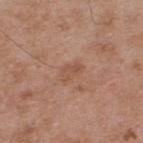  biopsy_status: not biopsied; imaged during a skin examination
  lesion_size:
    long_diameter_mm_approx: 3.0
  patient:
    sex: male
    age_approx: 55
  automated_metrics:
    area_mm2_approx: 4.0
    vs_skin_contrast_norm: 5.0
    border_irregularity_0_10: 3.0
    color_variation_0_10: 2.0
    peripheral_color_asymmetry: 1.0
    nevus_likeness_0_100: 0
  site: upper back
  lighting: white-light
  image:
    source: total-body photography crop
    field_of_view_mm: 15